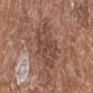No biopsy was performed on this lesion — it was imaged during a full skin examination and was not determined to be concerning.